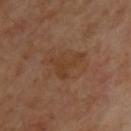<tbp_lesion>
<biopsy_status>not biopsied; imaged during a skin examination</biopsy_status>
<image>
  <source>total-body photography crop</source>
  <field_of_view_mm>15</field_of_view_mm>
</image>
<lesion_size>
  <long_diameter_mm_approx>4.0</long_diameter_mm_approx>
</lesion_size>
<site>back</site>
<automated_metrics>
  <area_mm2_approx>6.0</area_mm2_approx>
  <eccentricity>0.75</eccentricity>
  <shape_asymmetry>0.7</shape_asymmetry>
  <border_irregularity_0_10>7.5</border_irregularity_0_10>
  <color_variation_0_10>1.5</color_variation_0_10>
</automated_metrics>
</tbp_lesion>